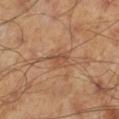This image is a 15 mm lesion crop taken from a total-body photograph.
On the left lower leg.
A male subject, aged 43–47.